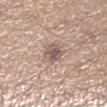Assessment: Captured during whole-body skin photography for melanoma surveillance; the lesion was not biopsied. Clinical summary: The total-body-photography lesion software estimated a within-lesion color-variation index near 4/10 and a peripheral color-asymmetry measure near 1.5. Approximately 3 mm at its widest. From the left lower leg. A region of skin cropped from a whole-body photographic capture, roughly 15 mm wide. A male patient roughly 40 years of age.